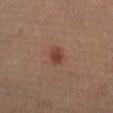{
  "image": {
    "source": "total-body photography crop",
    "field_of_view_mm": 15
  },
  "lighting": "cross-polarized",
  "automated_metrics": {
    "area_mm2_approx": 4.0,
    "eccentricity": 0.65,
    "shape_asymmetry": 0.2,
    "cielab_L": 40,
    "cielab_a": 21,
    "cielab_b": 28,
    "vs_skin_darker_L": 9.0,
    "vs_skin_contrast_norm": 8.0,
    "border_irregularity_0_10": 2.0,
    "color_variation_0_10": 2.5,
    "peripheral_color_asymmetry": 1.0,
    "nevus_likeness_0_100": 95
  },
  "site": "left leg",
  "patient": {
    "sex": "male",
    "age_approx": 50
  }
}